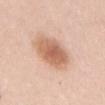Assessment:
Part of a total-body skin-imaging series; this lesion was reviewed on a skin check and was not flagged for biopsy.
Context:
A 15 mm close-up tile from a total-body photography series done for melanoma screening. Automated tile analysis of the lesion measured a border-irregularity index near 1.5/10, internal color variation of about 4.5 on a 0–10 scale, and peripheral color asymmetry of about 1. And it measured a classifier nevus-likeness of about 95/100. The lesion is located on the abdomen. This is a white-light tile. Measured at roughly 5.5 mm in maximum diameter. The subject is a female about 45 years old.